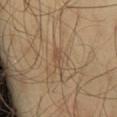<record>
<biopsy_status>not biopsied; imaged during a skin examination</biopsy_status>
<patient>
  <sex>male</sex>
  <age_approx>45</age_approx>
</patient>
<image>
  <source>total-body photography crop</source>
  <field_of_view_mm>15</field_of_view_mm>
</image>
<lighting>cross-polarized</lighting>
<site>right thigh</site>
<lesion_size>
  <long_diameter_mm_approx>3.0</long_diameter_mm_approx>
</lesion_size>
<automated_metrics>
  <area_mm2_approx>3.0</area_mm2_approx>
  <eccentricity>0.9</eccentricity>
  <shape_asymmetry>0.45</shape_asymmetry>
  <color_variation_0_10>0.5</color_variation_0_10>
  <peripheral_color_asymmetry>0.0</peripheral_color_asymmetry>
</automated_metrics>
</record>